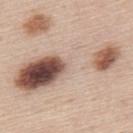The lesion was tiled from a total-body skin photograph and was not biopsied. A lesion tile, about 15 mm wide, cut from a 3D total-body photograph. Automated tile analysis of the lesion measured an area of roughly 55 mm² and an eccentricity of roughly 0.95. It also reported border irregularity of about 5 on a 0–10 scale and a peripheral color-asymmetry measure near 9. From the back. Captured under white-light illumination. A male subject, approximately 45 years of age.